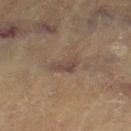Clinical impression:
This lesion was catalogued during total-body skin photography and was not selected for biopsy.
Acquisition and patient details:
The tile uses cross-polarized illumination. Automated tile analysis of the lesion measured an area of roughly 4 mm², an eccentricity of roughly 0.9, and a shape-asymmetry score of about 0.4 (0 = symmetric). And it measured a classifier nevus-likeness of about 0/100 and a lesion-detection confidence of about 70/100. The lesion is on the left thigh. Approximately 3.5 mm at its widest. The subject is a female aged 78 to 82. A 15 mm crop from a total-body photograph taken for skin-cancer surveillance.